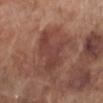Impression: Captured during whole-body skin photography for melanoma surveillance; the lesion was not biopsied. Acquisition and patient details: The lesion's longest dimension is about 6 mm. An algorithmic analysis of the crop reported a lesion area of about 17 mm² and two-axis asymmetry of about 0.4. And it measured border irregularity of about 6.5 on a 0–10 scale and radial color variation of about 1. It also reported a classifier nevus-likeness of about 5/100 and a lesion-detection confidence of about 100/100. A region of skin cropped from a whole-body photographic capture, roughly 15 mm wide. The patient is a male aged 68 to 72. Located on the left lower leg.